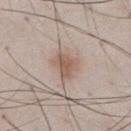| key | value |
|---|---|
| workup | imaged on a skin check; not biopsied |
| tile lighting | white-light illumination |
| site | the abdomen |
| imaging modality | ~15 mm crop, total-body skin-cancer survey |
| subject | male, roughly 50 years of age |
| automated lesion analysis | a nevus-likeness score of about 55/100 and lesion-presence confidence of about 100/100 |